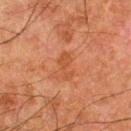Q: Is there a histopathology result?
A: catalogued during a skin exam; not biopsied
Q: What did automated image analysis measure?
A: a footprint of about 4 mm² and a shape-asymmetry score of about 0.4 (0 = symmetric)
Q: How large is the lesion?
A: ≈3.5 mm
Q: Patient demographics?
A: male, roughly 80 years of age
Q: How was the tile lit?
A: cross-polarized
Q: How was this image acquired?
A: 15 mm crop, total-body photography
Q: Where on the body is the lesion?
A: the left thigh